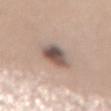Part of a total-body skin-imaging series; this lesion was reviewed on a skin check and was not flagged for biopsy. An algorithmic analysis of the crop reported a footprint of about 8.5 mm², a shape eccentricity near 0.8, and two-axis asymmetry of about 0.25. It also reported a lesion color around L≈53 a*≈15 b*≈22 in CIELAB, a lesion–skin lightness drop of about 15, and a normalized lesion–skin contrast near 10. The analysis additionally found a border-irregularity index near 2.5/10. Captured under white-light illumination. A female patient, aged 38 to 42. On the back. A 15 mm close-up tile from a total-body photography series done for melanoma screening.